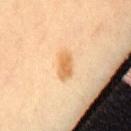Impression: The lesion was tiled from a total-body skin photograph and was not biopsied. Context: The recorded lesion diameter is about 3.5 mm. The total-body-photography lesion software estimated a shape eccentricity near 0.85 and a symmetry-axis asymmetry near 0.15. It also reported an automated nevus-likeness rating near 90 out of 100 and a lesion-detection confidence of about 100/100. A region of skin cropped from a whole-body photographic capture, roughly 15 mm wide. The patient is a female aged approximately 50.